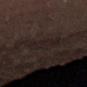{
  "biopsy_status": "not biopsied; imaged during a skin examination",
  "image": {
    "source": "total-body photography crop",
    "field_of_view_mm": 15
  },
  "site": "right thigh",
  "lesion_size": {
    "long_diameter_mm_approx": 4.0
  },
  "lighting": "cross-polarized",
  "patient": {
    "sex": "male",
    "age_approx": "85+"
  }
}